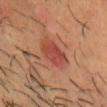<lesion>
<biopsy_status>not biopsied; imaged during a skin examination</biopsy_status>
</lesion>